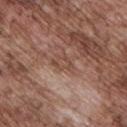{"biopsy_status": "not biopsied; imaged during a skin examination", "patient": {"sex": "male", "age_approx": 70}, "site": "front of the torso", "image": {"source": "total-body photography crop", "field_of_view_mm": 15}}